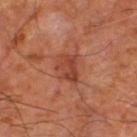This lesion was catalogued during total-body skin photography and was not selected for biopsy. On the right thigh. Captured under cross-polarized illumination. The patient is in their mid- to late 60s. The lesion-visualizer software estimated a lesion area of about 7 mm² and a shape eccentricity near 0.7. The analysis additionally found a mean CIELAB color near L≈43 a*≈28 b*≈31, a lesion–skin lightness drop of about 9, and a lesion-to-skin contrast of about 7 (normalized; higher = more distinct). The analysis additionally found a classifier nevus-likeness of about 5/100 and a lesion-detection confidence of about 100/100. This image is a 15 mm lesion crop taken from a total-body photograph.